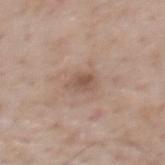biopsy status=no biopsy performed (imaged during a skin exam)
lesion size=about 3 mm
location=the mid back
image source=~15 mm tile from a whole-body skin photo
automated metrics=border irregularity of about 3 on a 0–10 scale, a within-lesion color-variation index near 3/10, and peripheral color asymmetry of about 1; a classifier nevus-likeness of about 15/100 and a detector confidence of about 100 out of 100 that the crop contains a lesion
subject=male, aged approximately 60
tile lighting=white-light illumination